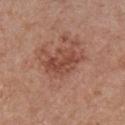follow-up: imaged on a skin check; not biopsied